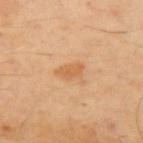A male patient about 50 years old.
From the left upper arm.
A 15 mm crop from a total-body photograph taken for skin-cancer surveillance.
Automated image analysis of the tile measured a nevus-likeness score of about 45/100 and a lesion-detection confidence of about 100/100.
The lesion's longest dimension is about 3 mm.
The tile uses cross-polarized illumination.